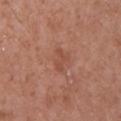This lesion was catalogued during total-body skin photography and was not selected for biopsy. The lesion is located on the front of the torso. A male patient in their 70s. A lesion tile, about 15 mm wide, cut from a 3D total-body photograph. About 2.5 mm across.